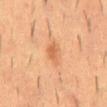notes = imaged on a skin check; not biopsied.